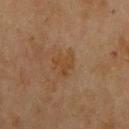Clinical impression:
The lesion was tiled from a total-body skin photograph and was not biopsied.
Acquisition and patient details:
A female subject, approximately 70 years of age. Imaged with cross-polarized lighting. From the right upper arm. Automated tile analysis of the lesion measured lesion-presence confidence of about 100/100. Cropped from a whole-body photographic skin survey; the tile spans about 15 mm.